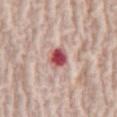workup = catalogued during a skin exam; not biopsied